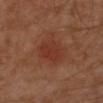<case>
<biopsy_status>not biopsied; imaged during a skin examination</biopsy_status>
<automated_metrics>
  <eccentricity>0.45</eccentricity>
  <shape_asymmetry>0.2</shape_asymmetry>
  <cielab_L>34</cielab_L>
  <cielab_a>25</cielab_a>
  <cielab_b>28</cielab_b>
  <nevus_likeness_0_100>95</nevus_likeness_0_100>
  <lesion_detection_confidence_0_100>100</lesion_detection_confidence_0_100>
</automated_metrics>
<lesion_size>
  <long_diameter_mm_approx>3.5</long_diameter_mm_approx>
</lesion_size>
<lighting>cross-polarized</lighting>
<image>
  <source>total-body photography crop</source>
  <field_of_view_mm>15</field_of_view_mm>
</image>
<patient>
  <sex>male</sex>
  <age_approx>30</age_approx>
</patient>
<site>arm</site>
</case>